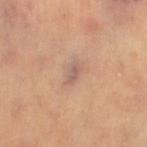Imaged during a routine full-body skin examination; the lesion was not biopsied and no histopathology is available.